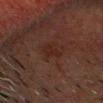notes: catalogued during a skin exam; not biopsied
image: 15 mm crop, total-body photography
location: the head or neck
illumination: cross-polarized
subject: male, in their 50s
size: ~3 mm (longest diameter)
TBP lesion metrics: a lesion area of about 3 mm² and an outline eccentricity of about 0.85 (0 = round, 1 = elongated); an average lesion color of about L≈18 a*≈17 b*≈19 (CIELAB) and a lesion–skin lightness drop of about 4; a border-irregularity rating of about 4/10 and a peripheral color-asymmetry measure near 0; a detector confidence of about 100 out of 100 that the crop contains a lesion